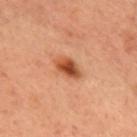Part of a total-body skin-imaging series; this lesion was reviewed on a skin check and was not flagged for biopsy.
A female patient in their mid-50s.
Automated tile analysis of the lesion measured a shape eccentricity near 0.8 and a symmetry-axis asymmetry near 0.15. The analysis additionally found border irregularity of about 1.5 on a 0–10 scale and internal color variation of about 5.5 on a 0–10 scale. The analysis additionally found a classifier nevus-likeness of about 100/100 and a detector confidence of about 100 out of 100 that the crop contains a lesion.
A 15 mm crop from a total-body photograph taken for skin-cancer surveillance.
The recorded lesion diameter is about 3.5 mm.
On the front of the torso.